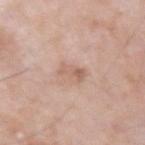A male subject, aged approximately 75.
Imaged with white-light lighting.
A 15 mm close-up extracted from a 3D total-body photography capture.
The lesion is located on the arm.
The recorded lesion diameter is about 2.5 mm.